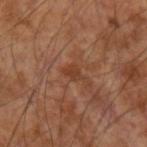Q: Was this lesion biopsied?
A: catalogued during a skin exam; not biopsied
Q: What did automated image analysis measure?
A: a footprint of about 3 mm², an outline eccentricity of about 0.8 (0 = round, 1 = elongated), and a shape-asymmetry score of about 0.4 (0 = symmetric); a border-irregularity index near 4.5/10, internal color variation of about 1.5 on a 0–10 scale, and peripheral color asymmetry of about 0.5; an automated nevus-likeness rating near 0 out of 100 and lesion-presence confidence of about 95/100
Q: Patient demographics?
A: male, approximately 55 years of age
Q: How was the tile lit?
A: cross-polarized illumination
Q: What is the imaging modality?
A: ~15 mm tile from a whole-body skin photo
Q: Lesion location?
A: the left forearm